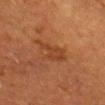  biopsy_status: not biopsied; imaged during a skin examination
  patient:
    sex: male
    age_approx: 75
  image:
    source: total-body photography crop
    field_of_view_mm: 15
  site: chest